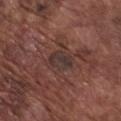Image and clinical context: On the chest. This is a white-light tile. The recorded lesion diameter is about 3.5 mm. Automated tile analysis of the lesion measured a footprint of about 5.5 mm², a shape eccentricity near 0.75, and two-axis asymmetry of about 0.25. The analysis additionally found an average lesion color of about L≈33 a*≈15 b*≈18 (CIELAB) and a lesion–skin lightness drop of about 6. The analysis additionally found a within-lesion color-variation index near 3/10 and peripheral color asymmetry of about 1. The software also gave lesion-presence confidence of about 75/100. This image is a 15 mm lesion crop taken from a total-body photograph. A male patient, aged around 75.